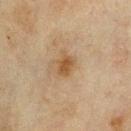Q: Was a biopsy performed?
A: no biopsy performed (imaged during a skin exam)
Q: How was this image acquired?
A: ~15 mm tile from a whole-body skin photo
Q: What lighting was used for the tile?
A: cross-polarized illumination
Q: What are the patient's age and sex?
A: male, about 70 years old
Q: How large is the lesion?
A: ~2.5 mm (longest diameter)
Q: What did automated image analysis measure?
A: a mean CIELAB color near L≈43 a*≈15 b*≈31 and roughly 8 lightness units darker than nearby skin; a border-irregularity rating of about 2.5/10 and a peripheral color-asymmetry measure near 0.5
Q: Where on the body is the lesion?
A: the chest